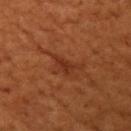Notes:
• notes — catalogued during a skin exam; not biopsied
• subject — female, aged approximately 80
• acquisition — 15 mm crop, total-body photography
• lesion size — about 2.5 mm
• lighting — cross-polarized
• site — the right upper arm
• automated lesion analysis — a lesion color around L≈24 a*≈21 b*≈26 in CIELAB and a normalized border contrast of about 6